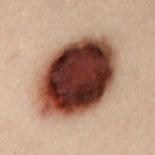Q: Was this lesion biopsied?
A: imaged on a skin check; not biopsied
Q: Lesion location?
A: the front of the torso
Q: What kind of image is this?
A: 15 mm crop, total-body photography
Q: Who is the patient?
A: female, in their 30s
Q: Automated lesion metrics?
A: a shape eccentricity near 0.7 and two-axis asymmetry of about 0.1; about 25 CIELAB-L* units darker than the surrounding skin and a normalized border contrast of about 19.5; border irregularity of about 1 on a 0–10 scale, a within-lesion color-variation index near 9/10, and peripheral color asymmetry of about 2.5; a nevus-likeness score of about 100/100 and a lesion-detection confidence of about 100/100